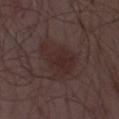Image and clinical context:
The lesion is on the chest. The tile uses white-light illumination. About 5.5 mm across. A region of skin cropped from a whole-body photographic capture, roughly 15 mm wide. A male subject, aged 53–57.